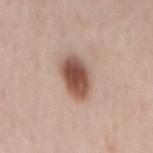notes: total-body-photography surveillance lesion; no biopsy